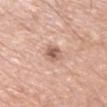Q: Is there a histopathology result?
A: imaged on a skin check; not biopsied
Q: What is the anatomic site?
A: the left upper arm
Q: What are the patient's age and sex?
A: male, roughly 75 years of age
Q: What is the lesion's diameter?
A: ≈2.5 mm
Q: Automated lesion metrics?
A: a border-irregularity index near 2/10 and a within-lesion color-variation index near 5.5/10; an automated nevus-likeness rating near 35 out of 100
Q: What kind of image is this?
A: total-body-photography crop, ~15 mm field of view
Q: What lighting was used for the tile?
A: white-light illumination